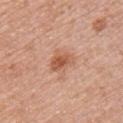Impression: Captured during whole-body skin photography for melanoma surveillance; the lesion was not biopsied. Context: The patient is a female aged 48 to 52. A region of skin cropped from a whole-body photographic capture, roughly 15 mm wide. The lesion is on the front of the torso.